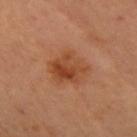biopsy_status: not biopsied; imaged during a skin examination
patient:
  sex: female
  age_approx: 40
lesion_size:
  long_diameter_mm_approx: 4.5
site: right forearm
lighting: cross-polarized
automated_metrics:
  border_irregularity_0_10: 3.0
  color_variation_0_10: 6.5
  peripheral_color_asymmetry: 2.5
  nevus_likeness_0_100: 60
  lesion_detection_confidence_0_100: 100
image:
  source: total-body photography crop
  field_of_view_mm: 15